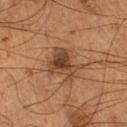Notes:
– patient: male, in their mid-50s
– size: ≈4.5 mm
– acquisition: ~15 mm crop, total-body skin-cancer survey
– body site: the right lower leg